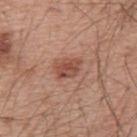Clinical impression:
The lesion was photographed on a routine skin check and not biopsied; there is no pathology result.
Image and clinical context:
A region of skin cropped from a whole-body photographic capture, roughly 15 mm wide. The lesion is located on the back. Automated image analysis of the tile measured a footprint of about 6 mm², an outline eccentricity of about 0.7 (0 = round, 1 = elongated), and a shape-asymmetry score of about 0.25 (0 = symmetric). And it measured a classifier nevus-likeness of about 75/100. The recorded lesion diameter is about 3 mm. A male patient aged 53 to 57. Captured under white-light illumination.